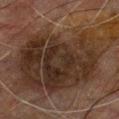  biopsy_status: not biopsied; imaged during a skin examination
  patient:
    sex: male
    age_approx: 65
  lesion_size:
    long_diameter_mm_approx: 16.0
  site: chest
  lighting: cross-polarized
  image:
    source: total-body photography crop
    field_of_view_mm: 15